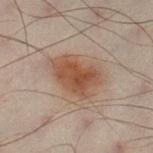follow-up: total-body-photography surveillance lesion; no biopsy
site: the left lower leg
imaging modality: total-body-photography crop, ~15 mm field of view
diameter: ~6 mm (longest diameter)
patient: male, aged approximately 50